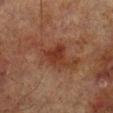follow-up: no biopsy performed (imaged during a skin exam)